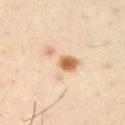The lesion was tiled from a total-body skin photograph and was not biopsied. The recorded lesion diameter is about 5.5 mm. This is a cross-polarized tile. A 15 mm close-up extracted from a 3D total-body photography capture. A male subject roughly 40 years of age. From the right upper arm.